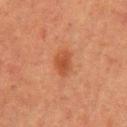Captured during whole-body skin photography for melanoma surveillance; the lesion was not biopsied. Cropped from a total-body skin-imaging series; the visible field is about 15 mm. Automated tile analysis of the lesion measured an automated nevus-likeness rating near 65 out of 100. The patient is a female in their mid-50s. Longest diameter approximately 3 mm. Located on the left upper arm. Imaged with cross-polarized lighting.